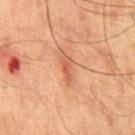Captured during whole-body skin photography for melanoma surveillance; the lesion was not biopsied.
The lesion is on the chest.
A 15 mm close-up extracted from a 3D total-body photography capture.
An algorithmic analysis of the crop reported a lesion area of about 3.5 mm², an outline eccentricity of about 0.9 (0 = round, 1 = elongated), and a shape-asymmetry score of about 0.3 (0 = symmetric).
A male subject, roughly 50 years of age.
Measured at roughly 3 mm in maximum diameter.